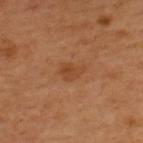lighting: cross-polarized
image:
  source: total-body photography crop
  field_of_view_mm: 15
site: upper back
lesion_size:
  long_diameter_mm_approx: 3.0
patient:
  sex: male
  age_approx: 50